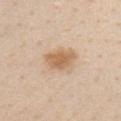Case summary:
• patient — male, in their 60s
• lesion diameter — about 4.5 mm
• lighting — white-light illumination
• location — the chest
• image-analysis metrics — an average lesion color of about L≈64 a*≈17 b*≈34 (CIELAB) and a normalized lesion–skin contrast near 7.5
• image source — ~15 mm tile from a whole-body skin photo